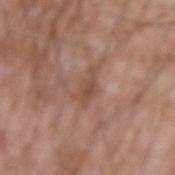{
  "biopsy_status": "not biopsied; imaged during a skin examination",
  "image": {
    "source": "total-body photography crop",
    "field_of_view_mm": 15
  },
  "site": "arm",
  "patient": {
    "sex": "male",
    "age_approx": 60
  }
}